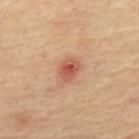Recorded during total-body skin imaging; not selected for excision or biopsy. The patient is a male roughly 65 years of age. From the abdomen. A 15 mm close-up extracted from a 3D total-body photography capture.